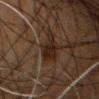Findings:
* subject · male, approximately 80 years of age
* automated metrics · a classifier nevus-likeness of about 5/100
* image source · 15 mm crop, total-body photography
* anatomic site · the chest
* tile lighting · cross-polarized illumination
* diameter · ≈3.5 mm